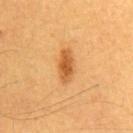Q: Was this lesion biopsied?
A: imaged on a skin check; not biopsied
Q: What lighting was used for the tile?
A: cross-polarized
Q: Patient demographics?
A: male, aged approximately 60
Q: What kind of image is this?
A: ~15 mm tile from a whole-body skin photo
Q: How large is the lesion?
A: about 4 mm
Q: What is the anatomic site?
A: the abdomen